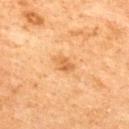Impression: Recorded during total-body skin imaging; not selected for excision or biopsy. Context: A 15 mm crop from a total-body photograph taken for skin-cancer surveillance. The lesion is on the back. A female patient aged 58 to 62. About 3 mm across. An algorithmic analysis of the crop reported a mean CIELAB color near L≈52 a*≈21 b*≈38 and about 8 CIELAB-L* units darker than the surrounding skin. The analysis additionally found a border-irregularity rating of about 2.5/10, internal color variation of about 2.5 on a 0–10 scale, and peripheral color asymmetry of about 1. This is a cross-polarized tile.